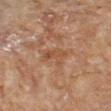Q: Was a biopsy performed?
A: total-body-photography surveillance lesion; no biopsy
Q: Lesion size?
A: about 2.5 mm
Q: Where on the body is the lesion?
A: the left forearm
Q: What is the imaging modality?
A: ~15 mm tile from a whole-body skin photo
Q: What did automated image analysis measure?
A: a footprint of about 5 mm² and an outline eccentricity of about 0.6 (0 = round, 1 = elongated); about 6 CIELAB-L* units darker than the surrounding skin; border irregularity of about 4.5 on a 0–10 scale and radial color variation of about 1
Q: Patient demographics?
A: female, roughly 80 years of age